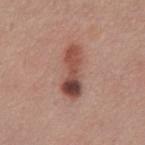This lesion was catalogued during total-body skin photography and was not selected for biopsy. An algorithmic analysis of the crop reported a lesion area of about 11 mm² and two-axis asymmetry of about 0.2. It also reported an average lesion color of about L≈48 a*≈23 b*≈26 (CIELAB), a lesion–skin lightness drop of about 14, and a lesion-to-skin contrast of about 9.5 (normalized; higher = more distinct). The software also gave a classifier nevus-likeness of about 45/100 and lesion-presence confidence of about 100/100. Captured under white-light illumination. A male subject aged approximately 55. A 15 mm close-up extracted from a 3D total-body photography capture. Located on the abdomen. Approximately 6 mm at its widest.